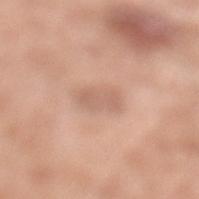| feature | finding |
|---|---|
| notes | no biopsy performed (imaged during a skin exam) |
| automated metrics | an area of roughly 5.5 mm², a shape eccentricity near 0.8, and a shape-asymmetry score of about 0.25 (0 = symmetric); an average lesion color of about L≈62 a*≈20 b*≈29 (CIELAB) and a normalized border contrast of about 5; a classifier nevus-likeness of about 0/100 and a detector confidence of about 100 out of 100 that the crop contains a lesion |
| patient | male, about 80 years old |
| image source | ~15 mm crop, total-body skin-cancer survey |
| tile lighting | white-light illumination |
| anatomic site | the left lower leg |
| lesion size | ≈3.5 mm |